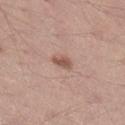biopsy status — no biopsy performed (imaged during a skin exam) | image source — 15 mm crop, total-body photography | tile lighting — white-light illumination | diameter — ~2.5 mm (longest diameter) | automated metrics — an average lesion color of about L≈54 a*≈20 b*≈26 (CIELAB), about 11 CIELAB-L* units darker than the surrounding skin, and a lesion-to-skin contrast of about 7.5 (normalized; higher = more distinct); border irregularity of about 3 on a 0–10 scale and a peripheral color-asymmetry measure near 0.5 | subject — male, about 35 years old | body site — the right lower leg.